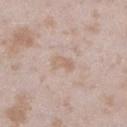Assessment:
Imaged during a routine full-body skin examination; the lesion was not biopsied and no histopathology is available.
Image and clinical context:
The subject is a female aged around 25. Captured under white-light illumination. From the left lower leg. A roughly 15 mm field-of-view crop from a total-body skin photograph.